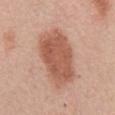Q: Was a biopsy performed?
A: total-body-photography surveillance lesion; no biopsy
Q: What did automated image analysis measure?
A: a lesion color around L≈57 a*≈23 b*≈30 in CIELAB, a lesion–skin lightness drop of about 12, and a lesion-to-skin contrast of about 8.5 (normalized; higher = more distinct)
Q: Patient demographics?
A: male, aged approximately 65
Q: Where on the body is the lesion?
A: the mid back
Q: How was this image acquired?
A: ~15 mm tile from a whole-body skin photo
Q: What lighting was used for the tile?
A: white-light illumination
Q: How large is the lesion?
A: ≈7.5 mm